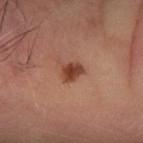<lesion>
  <biopsy_status>not biopsied; imaged during a skin examination</biopsy_status>
  <patient>
    <sex>male</sex>
    <age_approx>65</age_approx>
  </patient>
  <lighting>cross-polarized</lighting>
  <automated_metrics>
    <area_mm2_approx>5.0</area_mm2_approx>
    <eccentricity>0.5</eccentricity>
    <shape_asymmetry>0.3</shape_asymmetry>
    <vs_skin_darker_L>12.0</vs_skin_darker_L>
    <vs_skin_contrast_norm>9.5</vs_skin_contrast_norm>
  </automated_metrics>
  <site>left forearm</site>
  <image>
    <source>total-body photography crop</source>
    <field_of_view_mm>15</field_of_view_mm>
  </image>
  <lesion_size>
    <long_diameter_mm_approx>2.5</long_diameter_mm_approx>
  </lesion_size>
</lesion>